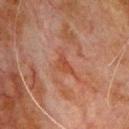workup = catalogued during a skin exam; not biopsied | patient = male, about 80 years old | size = ~2.5 mm (longest diameter) | anatomic site = the chest | tile lighting = cross-polarized | imaging modality = ~15 mm tile from a whole-body skin photo.